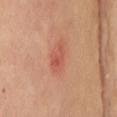Case summary:
- notes — catalogued during a skin exam; not biopsied
- tile lighting — cross-polarized illumination
- imaging modality — total-body-photography crop, ~15 mm field of view
- anatomic site — the chest